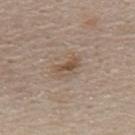Impression:
Imaged during a routine full-body skin examination; the lesion was not biopsied and no histopathology is available.